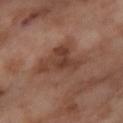Assessment:
Imaged during a routine full-body skin examination; the lesion was not biopsied and no histopathology is available.
Context:
This is a cross-polarized tile. The lesion is on the leg. Automated image analysis of the tile measured a detector confidence of about 100 out of 100 that the crop contains a lesion. The lesion's longest dimension is about 6 mm. A female patient, in their mid-50s. A close-up tile cropped from a whole-body skin photograph, about 15 mm across.